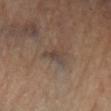The lesion was photographed on a routine skin check and not biopsied; there is no pathology result. Longest diameter approximately 3 mm. On the right lower leg. A female subject, in their 70s. A 15 mm close-up extracted from a 3D total-body photography capture. The tile uses cross-polarized illumination. Automated tile analysis of the lesion measured a footprint of about 3.5 mm², an eccentricity of roughly 0.85, and a symmetry-axis asymmetry near 0.45. The analysis additionally found a classifier nevus-likeness of about 0/100 and lesion-presence confidence of about 55/100.